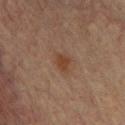{"biopsy_status": "not biopsied; imaged during a skin examination", "lighting": "cross-polarized", "site": "front of the torso", "image": {"source": "total-body photography crop", "field_of_view_mm": 15}, "patient": {"sex": "male", "age_approx": 60}}